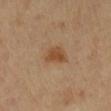Q: Was a biopsy performed?
A: total-body-photography surveillance lesion; no biopsy